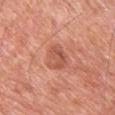<tbp_lesion>
<biopsy_status>not biopsied; imaged during a skin examination</biopsy_status>
<lighting>white-light</lighting>
<image>
  <source>total-body photography crop</source>
  <field_of_view_mm>15</field_of_view_mm>
</image>
<lesion_size>
  <long_diameter_mm_approx>3.5</long_diameter_mm_approx>
</lesion_size>
<patient>
  <sex>male</sex>
  <age_approx>60</age_approx>
</patient>
<automated_metrics>
  <area_mm2_approx>7.5</area_mm2_approx>
  <shape_asymmetry>0.25</shape_asymmetry>
  <cielab_L>56</cielab_L>
  <cielab_a>28</cielab_a>
  <cielab_b>32</cielab_b>
  <vs_skin_darker_L>9.0</vs_skin_darker_L>
  <vs_skin_contrast_norm>6.0</vs_skin_contrast_norm>
  <border_irregularity_0_10>2.5</border_irregularity_0_10>
  <color_variation_0_10>4.0</color_variation_0_10>
  <peripheral_color_asymmetry>1.5</peripheral_color_asymmetry>
  <nevus_likeness_0_100>0</nevus_likeness_0_100>
  <lesion_detection_confidence_0_100>100</lesion_detection_confidence_0_100>
</automated_metrics>
<site>chest</site>
</tbp_lesion>